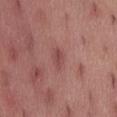Impression: Part of a total-body skin-imaging series; this lesion was reviewed on a skin check and was not flagged for biopsy. Acquisition and patient details: The lesion's longest dimension is about 2.5 mm. The lesion is located on the abdomen. Cropped from a whole-body photographic skin survey; the tile spans about 15 mm. The subject is a male about 70 years old. Imaged with white-light lighting.